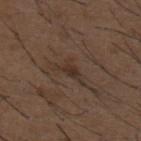notes: catalogued during a skin exam; not biopsied | subject: male, aged approximately 50 | image source: ~15 mm crop, total-body skin-cancer survey | illumination: white-light illumination | site: the upper back.